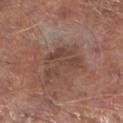Q: Patient demographics?
A: male, aged 63 to 67
Q: Lesion location?
A: the left lower leg
Q: What is the lesion's diameter?
A: ≈6 mm
Q: What is the imaging modality?
A: total-body-photography crop, ~15 mm field of view
Q: What did automated image analysis measure?
A: a lesion area of about 13 mm², an eccentricity of roughly 0.85, and a symmetry-axis asymmetry near 0.4; internal color variation of about 2.5 on a 0–10 scale and a peripheral color-asymmetry measure near 1; a nevus-likeness score of about 0/100 and a detector confidence of about 95 out of 100 that the crop contains a lesion
Q: How was the tile lit?
A: white-light illumination